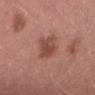{
  "biopsy_status": "not biopsied; imaged during a skin examination",
  "automated_metrics": {
    "area_mm2_approx": 8.0,
    "eccentricity": 0.8,
    "shape_asymmetry": 0.3,
    "cielab_L": 48,
    "cielab_a": 24,
    "cielab_b": 27,
    "vs_skin_contrast_norm": 7.5,
    "border_irregularity_0_10": 3.5,
    "color_variation_0_10": 3.0,
    "peripheral_color_asymmetry": 1.0
  },
  "lesion_size": {
    "long_diameter_mm_approx": 4.5
  },
  "site": "back",
  "lighting": "white-light",
  "image": {
    "source": "total-body photography crop",
    "field_of_view_mm": 15
  },
  "patient": {
    "sex": "male",
    "age_approx": 40
  }
}